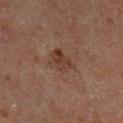Findings:
* acquisition · ~15 mm tile from a whole-body skin photo
* lesion diameter · about 3 mm
* patient · male, roughly 65 years of age
* anatomic site · the left lower leg
* tile lighting · cross-polarized